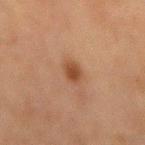{"lighting": "cross-polarized", "site": "back", "image": {"source": "total-body photography crop", "field_of_view_mm": 15}, "patient": {"sex": "male", "age_approx": 65}}